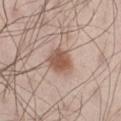This lesion was catalogued during total-body skin photography and was not selected for biopsy.
Located on the right thigh.
The tile uses white-light illumination.
Cropped from a total-body skin-imaging series; the visible field is about 15 mm.
A male subject, roughly 30 years of age.
The lesion-visualizer software estimated an eccentricity of roughly 0.3 and a symmetry-axis asymmetry near 0.15. And it measured a border-irregularity index near 1.5/10, a color-variation rating of about 3/10, and peripheral color asymmetry of about 1. And it measured a nevus-likeness score of about 95/100 and a lesion-detection confidence of about 100/100.